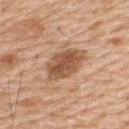Part of a total-body skin-imaging series; this lesion was reviewed on a skin check and was not flagged for biopsy. Longest diameter approximately 5 mm. A close-up tile cropped from a whole-body skin photograph, about 15 mm across. The lesion-visualizer software estimated an area of roughly 12 mm², an outline eccentricity of about 0.8 (0 = round, 1 = elongated), and two-axis asymmetry of about 0.15. The analysis additionally found an average lesion color of about L≈54 a*≈22 b*≈32 (CIELAB), a lesion–skin lightness drop of about 13, and a normalized border contrast of about 9. The analysis additionally found border irregularity of about 1.5 on a 0–10 scale, internal color variation of about 4.5 on a 0–10 scale, and radial color variation of about 1.5. It also reported a nevus-likeness score of about 85/100 and a detector confidence of about 100 out of 100 that the crop contains a lesion. From the upper back. The subject is a male aged around 60. Captured under white-light illumination.